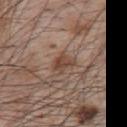• notes — no biopsy performed (imaged during a skin exam)
• subject — male, approximately 65 years of age
• imaging modality — ~15 mm crop, total-body skin-cancer survey
• size — about 3 mm
• TBP lesion metrics — an outline eccentricity of about 0.75 (0 = round, 1 = elongated) and two-axis asymmetry of about 0.3; an average lesion color of about L≈45 a*≈18 b*≈27 (CIELAB), about 9 CIELAB-L* units darker than the surrounding skin, and a normalized border contrast of about 7.5
• illumination — white-light
• site — the upper back